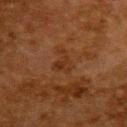Imaged during a routine full-body skin examination; the lesion was not biopsied and no histopathology is available.
A region of skin cropped from a whole-body photographic capture, roughly 15 mm wide.
Automated image analysis of the tile measured an average lesion color of about L≈27 a*≈19 b*≈29 (CIELAB), a lesion–skin lightness drop of about 5, and a normalized lesion–skin contrast near 5.5. The analysis additionally found radial color variation of about 1.
Captured under cross-polarized illumination.
Measured at roughly 3 mm in maximum diameter.
The lesion is on the upper back.
The subject is a female aged approximately 50.